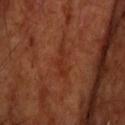Impression: Captured during whole-body skin photography for melanoma surveillance; the lesion was not biopsied. Acquisition and patient details: A close-up tile cropped from a whole-body skin photograph, about 15 mm across. Approximately 4.5 mm at its widest. The subject is a male about 70 years old. On the head or neck. Automated image analysis of the tile measured an eccentricity of roughly 0.95 and a symmetry-axis asymmetry near 0.4. The analysis additionally found lesion-presence confidence of about 95/100. Imaged with cross-polarized lighting.